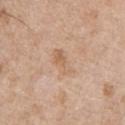The lesion was photographed on a routine skin check and not biopsied; there is no pathology result. A 15 mm crop from a total-body photograph taken for skin-cancer surveillance. The tile uses white-light illumination. About 4 mm across. The lesion is on the chest. A male subject roughly 65 years of age.